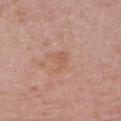Captured during whole-body skin photography for melanoma surveillance; the lesion was not biopsied. The lesion is located on the upper back. The subject is a male aged 38 to 42. About 3 mm across. Cropped from a total-body skin-imaging series; the visible field is about 15 mm.